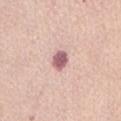biopsy status = total-body-photography surveillance lesion; no biopsy
size = about 2.5 mm
patient = female, aged approximately 55
acquisition = 15 mm crop, total-body photography
anatomic site = the abdomen